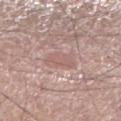Findings:
• notes: no biopsy performed (imaged during a skin exam)
• subject: male, aged around 50
• imaging modality: ~15 mm tile from a whole-body skin photo
• lesion size: about 3.5 mm
• site: the right lower leg
• image-analysis metrics: a mean CIELAB color near L≈59 a*≈19 b*≈22, roughly 7 lightness units darker than nearby skin, and a lesion-to-skin contrast of about 4.5 (normalized; higher = more distinct); a border-irregularity rating of about 2/10; a classifier nevus-likeness of about 0/100 and lesion-presence confidence of about 70/100